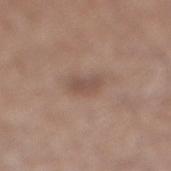The lesion was photographed on a routine skin check and not biopsied; there is no pathology result.
Located on the right lower leg.
A male patient, in their mid- to late 60s.
A 15 mm crop from a total-body photograph taken for skin-cancer surveillance.
This is a white-light tile.
Longest diameter approximately 2.5 mm.